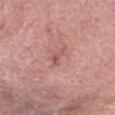Clinical impression:
This lesion was catalogued during total-body skin photography and was not selected for biopsy.
Context:
On the head or neck. This is a white-light tile. An algorithmic analysis of the crop reported an eccentricity of roughly 0.9 and a symmetry-axis asymmetry near 0.5. And it measured a border-irregularity index near 5/10, a color-variation rating of about 0/10, and peripheral color asymmetry of about 0. Longest diameter approximately 3 mm. A 15 mm crop from a total-body photograph taken for skin-cancer surveillance. A female patient in their mid-80s or older.